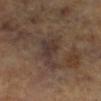The lesion was tiled from a total-body skin photograph and was not biopsied.
The patient is a female about 65 years old.
Cropped from a total-body skin-imaging series; the visible field is about 15 mm.
This is a cross-polarized tile.
The lesion is located on the left leg.
The recorded lesion diameter is about 6 mm.